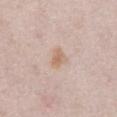biopsy_status: not biopsied; imaged during a skin examination
image:
  source: total-body photography crop
  field_of_view_mm: 15
lighting: white-light
lesion_size:
  long_diameter_mm_approx: 3.0
site: abdomen
automated_metrics:
  cielab_L: 65
  cielab_a: 16
  cielab_b: 30
  nevus_likeness_0_100: 70
  lesion_detection_confidence_0_100: 100
patient:
  sex: female
  age_approx: 50